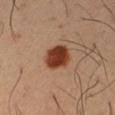Impression: The lesion was photographed on a routine skin check and not biopsied; there is no pathology result. Context: A 15 mm close-up tile from a total-body photography series done for melanoma screening. Longest diameter approximately 3.5 mm. On the right upper arm. The patient is a male aged 38–42. Captured under cross-polarized illumination.